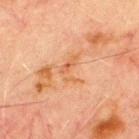follow-up: imaged on a skin check; not biopsied | acquisition: 15 mm crop, total-body photography | body site: the chest | patient: male, aged around 70.